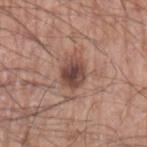follow-up: imaged on a skin check; not biopsied | imaging modality: ~15 mm tile from a whole-body skin photo | tile lighting: white-light | size: about 4 mm | image-analysis metrics: an area of roughly 8 mm², an outline eccentricity of about 0.65 (0 = round, 1 = elongated), and a symmetry-axis asymmetry near 0.2; a border-irregularity index near 2/10 and a color-variation rating of about 5/10 | subject: male, aged 68–72 | site: the left upper arm.